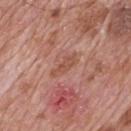Captured during whole-body skin photography for melanoma surveillance; the lesion was not biopsied.
A male patient aged approximately 70.
A region of skin cropped from a whole-body photographic capture, roughly 15 mm wide.
About 4 mm across.
On the mid back.